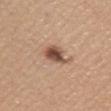biopsy status: imaged on a skin check; not biopsied
site: the left upper arm
patient: female, aged 58–62
lesion size: ≈3.5 mm
image: ~15 mm tile from a whole-body skin photo
lighting: white-light illumination